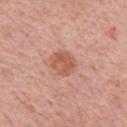The lesion was tiled from a total-body skin photograph and was not biopsied.
Located on the left upper arm.
Cropped from a total-body skin-imaging series; the visible field is about 15 mm.
The tile uses white-light illumination.
A female patient, aged 63 to 67.
Measured at roughly 3 mm in maximum diameter.